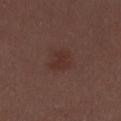This lesion was catalogued during total-body skin photography and was not selected for biopsy. The subject is a male aged around 30. This image is a 15 mm lesion crop taken from a total-body photograph. Located on the mid back.